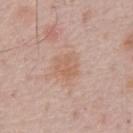<tbp_lesion>
<biopsy_status>not biopsied; imaged during a skin examination</biopsy_status>
<image>
  <source>total-body photography crop</source>
  <field_of_view_mm>15</field_of_view_mm>
</image>
<site>chest</site>
<lesion_size>
  <long_diameter_mm_approx>3.0</long_diameter_mm_approx>
</lesion_size>
<lighting>white-light</lighting>
<patient>
  <sex>male</sex>
  <age_approx>70</age_approx>
</patient>
<automated_metrics>
  <area_mm2_approx>7.5</area_mm2_approx>
  <eccentricity>0.45</eccentricity>
  <shape_asymmetry>0.25</shape_asymmetry>
  <cielab_L>61</cielab_L>
  <cielab_a>20</cielab_a>
  <cielab_b>29</cielab_b>
  <border_irregularity_0_10>2.5</border_irregularity_0_10>
  <color_variation_0_10>2.5</color_variation_0_10>
  <peripheral_color_asymmetry>1.0</peripheral_color_asymmetry>
  <nevus_likeness_0_100>30</nevus_likeness_0_100>
</automated_metrics>
</tbp_lesion>